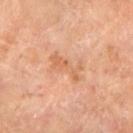Clinical impression:
Captured during whole-body skin photography for melanoma surveillance; the lesion was not biopsied.
Clinical summary:
The lesion is on the leg. A 15 mm crop from a total-body photograph taken for skin-cancer surveillance. Measured at roughly 4.5 mm in maximum diameter. The tile uses cross-polarized illumination. The subject is a female in their 70s.